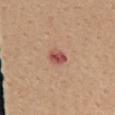biopsy status=no biopsy performed (imaged during a skin exam)
lesion size=~2.5 mm (longest diameter)
site=the mid back
lighting=white-light
patient=female, in their mid- to late 30s
imaging modality=~15 mm tile from a whole-body skin photo